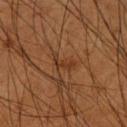| field | value |
|---|---|
| notes | catalogued during a skin exam; not biopsied |
| patient | male, approximately 60 years of age |
| body site | the right upper arm |
| imaging modality | ~15 mm crop, total-body skin-cancer survey |